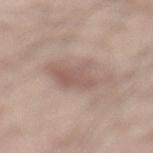| feature | finding |
|---|---|
| follow-up | catalogued during a skin exam; not biopsied |
| location | the left lower leg |
| imaging modality | total-body-photography crop, ~15 mm field of view |
| image-analysis metrics | a footprint of about 13 mm², an outline eccentricity of about 0.7 (0 = round, 1 = elongated), and a shape-asymmetry score of about 0.3 (0 = symmetric) |
| patient | male, about 35 years old |